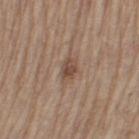Recorded during total-body skin imaging; not selected for excision or biopsy.
A male subject aged around 70.
The lesion is on the left thigh.
A 15 mm close-up tile from a total-body photography series done for melanoma screening.